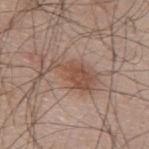Captured during whole-body skin photography for melanoma surveillance; the lesion was not biopsied.
About 6 mm across.
A lesion tile, about 15 mm wide, cut from a 3D total-body photograph.
A male patient aged approximately 45.
This is a white-light tile.
Automated tile analysis of the lesion measured an outline eccentricity of about 0.9 (0 = round, 1 = elongated) and two-axis asymmetry of about 0.55.
From the upper back.